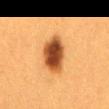Q: Was this lesion biopsied?
A: total-body-photography surveillance lesion; no biopsy
Q: How was the tile lit?
A: cross-polarized illumination
Q: How large is the lesion?
A: ≈5 mm
Q: What is the imaging modality?
A: total-body-photography crop, ~15 mm field of view
Q: Who is the patient?
A: female, approximately 40 years of age
Q: What is the anatomic site?
A: the mid back